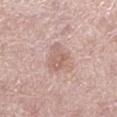| feature | finding |
|---|---|
| follow-up | no biopsy performed (imaged during a skin exam) |
| location | the left lower leg |
| tile lighting | white-light illumination |
| acquisition | 15 mm crop, total-body photography |
| lesion size | about 3.5 mm |
| automated lesion analysis | a border-irregularity rating of about 3.5/10, a within-lesion color-variation index near 3/10, and radial color variation of about 1; a classifier nevus-likeness of about 0/100 and lesion-presence confidence of about 100/100 |
| patient | male, about 60 years old |